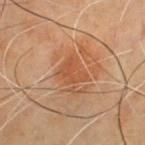Q: Was this lesion biopsied?
A: catalogued during a skin exam; not biopsied
Q: Lesion size?
A: ~3 mm (longest diameter)
Q: Patient demographics?
A: male, aged 53 to 57
Q: What did automated image analysis measure?
A: a mean CIELAB color near L≈42 a*≈21 b*≈31 and a lesion–skin lightness drop of about 5
Q: What is the anatomic site?
A: the front of the torso
Q: What kind of image is this?
A: ~15 mm tile from a whole-body skin photo